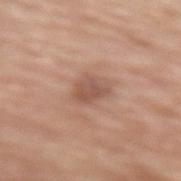Context: A male subject approximately 70 years of age. The lesion's longest dimension is about 3.5 mm. This is a white-light tile. An algorithmic analysis of the crop reported an area of roughly 6 mm² and an eccentricity of roughly 0.7. And it measured an average lesion color of about L≈54 a*≈20 b*≈27 (CIELAB). From the lower back. A region of skin cropped from a whole-body photographic capture, roughly 15 mm wide.